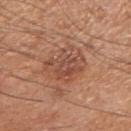biopsy status: total-body-photography surveillance lesion; no biopsy
diameter: ~5 mm (longest diameter)
anatomic site: the arm
subject: male, aged approximately 65
image: ~15 mm crop, total-body skin-cancer survey
image-analysis metrics: border irregularity of about 4 on a 0–10 scale, a within-lesion color-variation index near 4.5/10, and a peripheral color-asymmetry measure near 1.5; lesion-presence confidence of about 100/100